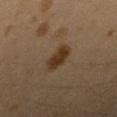This lesion was catalogued during total-body skin photography and was not selected for biopsy.